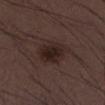  biopsy_status: not biopsied; imaged during a skin examination
  image:
    source: total-body photography crop
    field_of_view_mm: 15
  patient:
    sex: male
    age_approx: 50
  automated_metrics:
    cielab_L: 22
    cielab_a: 14
    cielab_b: 16
    vs_skin_darker_L: 8.0
    vs_skin_contrast_norm: 9.0
    border_irregularity_0_10: 1.5
    color_variation_0_10: 3.5
    peripheral_color_asymmetry: 1.0
    nevus_likeness_0_100: 65
    lesion_detection_confidence_0_100: 100
  lighting: white-light
  lesion_size:
    long_diameter_mm_approx: 3.5
  site: left lower leg